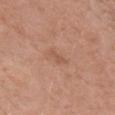Case summary:
– notes: total-body-photography surveillance lesion; no biopsy
– diameter: ~2.5 mm (longest diameter)
– tile lighting: white-light illumination
– anatomic site: the chest
– subject: female, about 60 years old
– image: total-body-photography crop, ~15 mm field of view